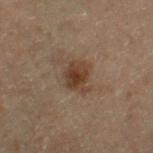{
  "biopsy_status": "not biopsied; imaged during a skin examination",
  "patient": {
    "sex": "male",
    "age_approx": 70
  },
  "site": "right thigh",
  "lighting": "cross-polarized",
  "image": {
    "source": "total-body photography crop",
    "field_of_view_mm": 15
  }
}